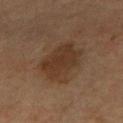Impression: Recorded during total-body skin imaging; not selected for excision or biopsy. Clinical summary: A 15 mm crop from a total-body photograph taken for skin-cancer surveillance. The tile uses cross-polarized illumination. A female patient roughly 40 years of age. The lesion's longest dimension is about 5 mm. On the left forearm. An algorithmic analysis of the crop reported a footprint of about 17 mm², an outline eccentricity of about 0.5 (0 = round, 1 = elongated), and two-axis asymmetry of about 0.2. The software also gave a border-irregularity index near 2.5/10, a color-variation rating of about 2.5/10, and a peripheral color-asymmetry measure near 1.